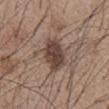Q: Was this lesion biopsied?
A: total-body-photography surveillance lesion; no biopsy
Q: How large is the lesion?
A: about 5 mm
Q: How was this image acquired?
A: ~15 mm crop, total-body skin-cancer survey
Q: What did automated image analysis measure?
A: a mean CIELAB color near L≈43 a*≈16 b*≈23, about 12 CIELAB-L* units darker than the surrounding skin, and a normalized lesion–skin contrast near 10
Q: Patient demographics?
A: male, approximately 55 years of age
Q: Where on the body is the lesion?
A: the front of the torso